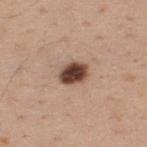<case>
<biopsy_status>not biopsied; imaged during a skin examination</biopsy_status>
<image>
  <source>total-body photography crop</source>
  <field_of_view_mm>15</field_of_view_mm>
</image>
<site>upper back</site>
<automated_metrics>
  <cielab_L>44</cielab_L>
  <cielab_a>20</cielab_a>
  <cielab_b>26</cielab_b>
  <vs_skin_darker_L>21.0</vs_skin_darker_L>
  <vs_skin_contrast_norm>14.5</vs_skin_contrast_norm>
  <nevus_likeness_0_100>95</nevus_likeness_0_100>
  <lesion_detection_confidence_0_100>100</lesion_detection_confidence_0_100>
</automated_metrics>
<patient>
  <sex>male</sex>
  <age_approx>40</age_approx>
</patient>
<lighting>white-light</lighting>
</case>